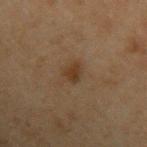<lesion>
<site>left upper arm</site>
<lighting>cross-polarized</lighting>
<automated_metrics>
  <area_mm2_approx>3.5</area_mm2_approx>
</automated_metrics>
<patient>
  <sex>male</sex>
  <age_approx>70</age_approx>
</patient>
<image>
  <source>total-body photography crop</source>
  <field_of_view_mm>15</field_of_view_mm>
</image>
<lesion_size>
  <long_diameter_mm_approx>3.0</long_diameter_mm_approx>
</lesion_size>
</lesion>